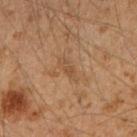From the right upper arm.
A 15 mm crop from a total-body photograph taken for skin-cancer surveillance.
The lesion's longest dimension is about 3 mm.
Captured under cross-polarized illumination.
The patient is a male aged approximately 50.